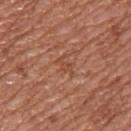Q: Was a biopsy performed?
A: catalogued during a skin exam; not biopsied
Q: Who is the patient?
A: male, in their mid- to late 50s
Q: How was this image acquired?
A: ~15 mm crop, total-body skin-cancer survey
Q: What did automated image analysis measure?
A: an area of roughly 3 mm², an outline eccentricity of about 0.85 (0 = round, 1 = elongated), and a symmetry-axis asymmetry near 0.55; a lesion–skin lightness drop of about 6 and a normalized lesion–skin contrast near 5; a nevus-likeness score of about 0/100 and a lesion-detection confidence of about 90/100
Q: How was the tile lit?
A: white-light illumination
Q: Lesion size?
A: ~2.5 mm (longest diameter)
Q: Lesion location?
A: the upper back